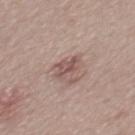follow-up: imaged on a skin check; not biopsied